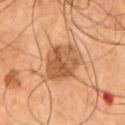image: 15 mm crop, total-body photography | location: the chest | patient: male, roughly 55 years of age.